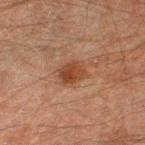notes: catalogued during a skin exam; not biopsied.